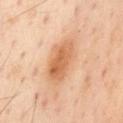Context: A 15 mm close-up extracted from a 3D total-body photography capture. Measured at roughly 5.5 mm in maximum diameter. A male patient aged around 55. On the chest. The tile uses cross-polarized illumination. Automated tile analysis of the lesion measured a shape eccentricity near 0.85 and a symmetry-axis asymmetry near 0.15. The analysis additionally found roughly 11 lightness units darker than nearby skin and a lesion-to-skin contrast of about 7.5 (normalized; higher = more distinct). The analysis additionally found a border-irregularity rating of about 2/10 and radial color variation of about 1.5. The software also gave an automated nevus-likeness rating near 90 out of 100 and a lesion-detection confidence of about 100/100.